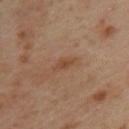Assessment: No biopsy was performed on this lesion — it was imaged during a full skin examination and was not determined to be concerning. Context: The lesion is located on the upper back. A female patient, about 40 years old. This is a cross-polarized tile. Longest diameter approximately 3 mm. A 15 mm close-up extracted from a 3D total-body photography capture.